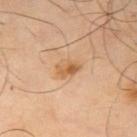Assessment:
Captured during whole-body skin photography for melanoma surveillance; the lesion was not biopsied.
Acquisition and patient details:
A 15 mm close-up tile from a total-body photography series done for melanoma screening. Longest diameter approximately 3 mm. This is a cross-polarized tile. The subject is a male aged approximately 65. On the right upper arm.